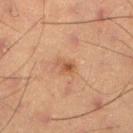{"biopsy_status": "not biopsied; imaged during a skin examination", "image": {"source": "total-body photography crop", "field_of_view_mm": 15}, "site": "right lower leg", "patient": {"sex": "male", "age_approx": 55}, "lighting": "cross-polarized"}